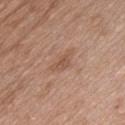Assessment: The lesion was tiled from a total-body skin photograph and was not biopsied. Context: An algorithmic analysis of the crop reported a footprint of about 3.5 mm² and a shape-asymmetry score of about 0.45 (0 = symmetric). The analysis additionally found an average lesion color of about L≈53 a*≈20 b*≈29 (CIELAB) and about 7 CIELAB-L* units darker than the surrounding skin. Approximately 3 mm at its widest. The lesion is located on the chest. A male patient, aged approximately 50. This image is a 15 mm lesion crop taken from a total-body photograph. This is a white-light tile.